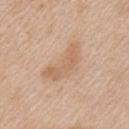Captured during whole-body skin photography for melanoma surveillance; the lesion was not biopsied. Longest diameter approximately 5.5 mm. A female patient, approximately 40 years of age. A roughly 15 mm field-of-view crop from a total-body skin photograph. The lesion is located on the arm. Captured under white-light illumination.